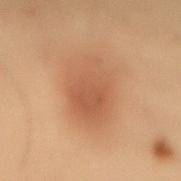{"lighting": "cross-polarized", "image": {"source": "total-body photography crop", "field_of_view_mm": 15}, "automated_metrics": {"area_mm2_approx": 21.0, "eccentricity": 0.75, "shape_asymmetry": 0.1, "border_irregularity_0_10": 2.0, "color_variation_0_10": 3.5, "peripheral_color_asymmetry": 1.0}, "patient": {"sex": "male", "age_approx": 55}, "site": "lower back"}